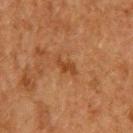  patient:
    sex: male
    age_approx: 50
  site: upper back
  lighting: cross-polarized
  image:
    source: total-body photography crop
    field_of_view_mm: 15
  lesion_size:
    long_diameter_mm_approx: 3.0